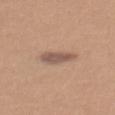workup: imaged on a skin check; not biopsied
subject: female, approximately 40 years of age
location: the back
image-analysis metrics: a mean CIELAB color near L≈54 a*≈18 b*≈25
imaging modality: 15 mm crop, total-body photography